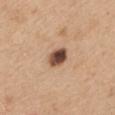Findings:
- workup — imaged on a skin check; not biopsied
- acquisition — 15 mm crop, total-body photography
- site — the mid back
- lighting — white-light
- patient — female, aged approximately 45
- size — ≈3 mm